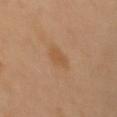biopsy status=no biopsy performed (imaged during a skin exam); illumination=cross-polarized; automated lesion analysis=a shape eccentricity near 0.75 and a symmetry-axis asymmetry near 0.2; subject=female, aged approximately 55; size=≈3 mm; anatomic site=the left upper arm; acquisition=total-body-photography crop, ~15 mm field of view.